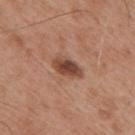Captured during whole-body skin photography for melanoma surveillance; the lesion was not biopsied. This is a white-light tile. Automated image analysis of the tile measured a footprint of about 6.5 mm² and an eccentricity of roughly 0.85. The software also gave a lesion color around L≈45 a*≈22 b*≈28 in CIELAB. It also reported border irregularity of about 2 on a 0–10 scale, a within-lesion color-variation index near 4/10, and a peripheral color-asymmetry measure near 1. A 15 mm close-up extracted from a 3D total-body photography capture. The recorded lesion diameter is about 3.5 mm. The patient is a male roughly 55 years of age. The lesion is on the mid back.